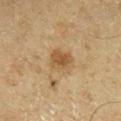Q: Is there a histopathology result?
A: no biopsy performed (imaged during a skin exam)
Q: Lesion location?
A: the right lower leg
Q: What are the patient's age and sex?
A: male, aged approximately 65
Q: What is the lesion's diameter?
A: ≈3 mm
Q: What kind of image is this?
A: total-body-photography crop, ~15 mm field of view
Q: What did automated image analysis measure?
A: an automated nevus-likeness rating near 65 out of 100 and a lesion-detection confidence of about 100/100
Q: What lighting was used for the tile?
A: cross-polarized